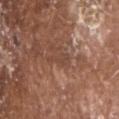Recorded during total-body skin imaging; not selected for excision or biopsy.
From the head or neck.
Automated image analysis of the tile measured an average lesion color of about L≈44 a*≈21 b*≈27 (CIELAB), about 6 CIELAB-L* units darker than the surrounding skin, and a lesion-to-skin contrast of about 4.5 (normalized; higher = more distinct). The software also gave a nevus-likeness score of about 0/100.
A male subject, approximately 80 years of age.
A 15 mm crop from a total-body photograph taken for skin-cancer surveillance.
The tile uses white-light illumination.